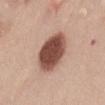Impression:
No biopsy was performed on this lesion — it was imaged during a full skin examination and was not determined to be concerning.
Acquisition and patient details:
This is a white-light tile. The lesion-visualizer software estimated a mean CIELAB color near L≈49 a*≈22 b*≈26, about 20 CIELAB-L* units darker than the surrounding skin, and a normalized lesion–skin contrast near 13. And it measured a within-lesion color-variation index near 5/10 and radial color variation of about 1.5. It also reported a nevus-likeness score of about 100/100 and a lesion-detection confidence of about 100/100. On the chest. A region of skin cropped from a whole-body photographic capture, roughly 15 mm wide. The subject is a male aged 48 to 52.